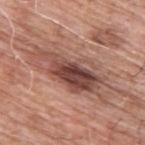This is a white-light tile.
This image is a 15 mm lesion crop taken from a total-body photograph.
An algorithmic analysis of the crop reported two-axis asymmetry of about 0.35. The software also gave about 14 CIELAB-L* units darker than the surrounding skin and a normalized lesion–skin contrast near 10.5.
The subject is a male about 60 years old.
About 7.5 mm across.
The lesion is located on the upper back.